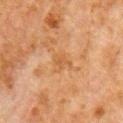| key | value |
|---|---|
| biopsy status | total-body-photography surveillance lesion; no biopsy |
| lighting | cross-polarized illumination |
| automated metrics | an area of roughly 3.5 mm², an outline eccentricity of about 0.8 (0 = round, 1 = elongated), and a shape-asymmetry score of about 0.35 (0 = symmetric); radial color variation of about 0.5 |
| subject | male, aged 78 to 82 |
| acquisition | ~15 mm crop, total-body skin-cancer survey |
| lesion size | ~3 mm (longest diameter) |
| body site | the right upper arm |